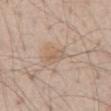Findings:
• workup — total-body-photography surveillance lesion; no biopsy
• acquisition — ~15 mm tile from a whole-body skin photo
• site — the abdomen
• lighting — white-light
• patient — male, roughly 70 years of age
• automated lesion analysis — an automated nevus-likeness rating near 10 out of 100 and a lesion-detection confidence of about 100/100
• lesion diameter — ~2.5 mm (longest diameter)